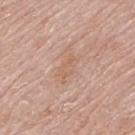Clinical impression: Part of a total-body skin-imaging series; this lesion was reviewed on a skin check and was not flagged for biopsy. Acquisition and patient details: A 15 mm close-up tile from a total-body photography series done for melanoma screening. The patient is a male aged 78 to 82. The lesion is on the upper back. Captured under white-light illumination. Automated tile analysis of the lesion measured a lesion area of about 4 mm² and a shape-asymmetry score of about 0.35 (0 = symmetric). The analysis additionally found an average lesion color of about L≈61 a*≈20 b*≈30 (CIELAB), a lesion–skin lightness drop of about 6, and a normalized border contrast of about 4.5. Measured at roughly 3.5 mm in maximum diameter.